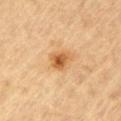notes: no biopsy performed (imaged during a skin exam); location: the arm; patient: male, aged 83 or older; image source: ~15 mm tile from a whole-body skin photo; lighting: cross-polarized.